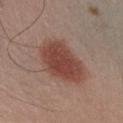biopsy status: no biopsy performed (imaged during a skin exam) | lesion size: about 6.5 mm | automated lesion analysis: a lesion area of about 20 mm²; a nevus-likeness score of about 100/100 | imaging modality: ~15 mm tile from a whole-body skin photo | patient: male, about 55 years old | location: the chest | illumination: white-light illumination.